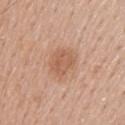Notes:
• follow-up: total-body-photography surveillance lesion; no biopsy
• imaging modality: 15 mm crop, total-body photography
• patient: male, aged 58–62
• tile lighting: white-light
• automated metrics: a footprint of about 7.5 mm², an eccentricity of roughly 0.45, and a symmetry-axis asymmetry near 0.2; a lesion color around L≈58 a*≈22 b*≈32 in CIELAB, roughly 8 lightness units darker than nearby skin, and a normalized border contrast of about 6
• anatomic site: the back